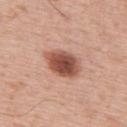Recorded during total-body skin imaging; not selected for excision or biopsy.
Approximately 5 mm at its widest.
Located on the upper back.
A male subject aged around 65.
A region of skin cropped from a whole-body photographic capture, roughly 15 mm wide.
The lesion-visualizer software estimated a lesion area of about 11 mm² and an eccentricity of roughly 0.75. It also reported about 17 CIELAB-L* units darker than the surrounding skin and a lesion-to-skin contrast of about 11 (normalized; higher = more distinct).